From the left lower leg. A lesion tile, about 15 mm wide, cut from a 3D total-body photograph. The subject is a male about 45 years old: 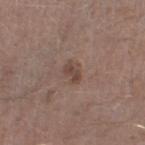{"lesion_size": {"long_diameter_mm_approx": 2.5}, "lighting": "white-light", "diagnosis": {"histopathology": "seborrheic keratosis", "malignancy": "benign", "taxonomic_path": ["Benign", "Benign epidermal proliferations", "Seborrheic keratosis"]}}